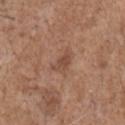Captured during whole-body skin photography for melanoma surveillance; the lesion was not biopsied.
The subject is a male aged 68–72.
A 15 mm crop from a total-body photograph taken for skin-cancer surveillance.
From the arm.